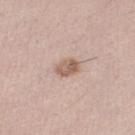Clinical impression: This lesion was catalogued during total-body skin photography and was not selected for biopsy. Background: A female patient, aged 38 to 42. From the leg. The lesion's longest dimension is about 3 mm. A region of skin cropped from a whole-body photographic capture, roughly 15 mm wide. An algorithmic analysis of the crop reported a lesion area of about 5 mm², an outline eccentricity of about 0.75 (0 = round, 1 = elongated), and two-axis asymmetry of about 0.2. The analysis additionally found a mean CIELAB color near L≈60 a*≈18 b*≈27, roughly 11 lightness units darker than nearby skin, and a normalized border contrast of about 7.5. The analysis additionally found a border-irregularity index near 1.5/10 and internal color variation of about 4.5 on a 0–10 scale.